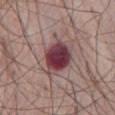Captured during whole-body skin photography for melanoma surveillance; the lesion was not biopsied.
Captured under white-light illumination.
A close-up tile cropped from a whole-body skin photograph, about 15 mm across.
About 5 mm across.
The subject is a male aged around 65.
The lesion is located on the abdomen.